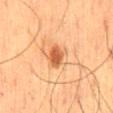{
  "biopsy_status": "not biopsied; imaged during a skin examination",
  "patient": {
    "sex": "male",
    "age_approx": 60
  },
  "lighting": "cross-polarized",
  "automated_metrics": {
    "area_mm2_approx": 5.0,
    "shape_asymmetry": 0.25,
    "vs_skin_darker_L": 12.0,
    "vs_skin_contrast_norm": 9.0,
    "nevus_likeness_0_100": 95
  },
  "lesion_size": {
    "long_diameter_mm_approx": 3.0
  },
  "site": "mid back",
  "image": {
    "source": "total-body photography crop",
    "field_of_view_mm": 15
  }
}